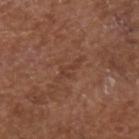Clinical impression:
No biopsy was performed on this lesion — it was imaged during a full skin examination and was not determined to be concerning.
Background:
Measured at roughly 4 mm in maximum diameter. From the head or neck. A roughly 15 mm field-of-view crop from a total-body skin photograph. A male patient aged around 80. This is a white-light tile.